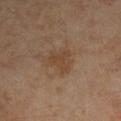Recorded during total-body skin imaging; not selected for excision or biopsy. A male patient, aged approximately 55. A close-up tile cropped from a whole-body skin photograph, about 15 mm across. Located on the right upper arm. The tile uses cross-polarized illumination. The lesion's longest dimension is about 3 mm.